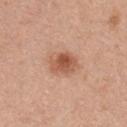Imaged during a routine full-body skin examination; the lesion was not biopsied and no histopathology is available. About 3.5 mm across. This is a white-light tile. From the chest. A lesion tile, about 15 mm wide, cut from a 3D total-body photograph. A female subject, roughly 30 years of age.